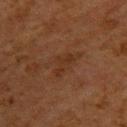| feature | finding |
|---|---|
| workup | catalogued during a skin exam; not biopsied |
| image | 15 mm crop, total-body photography |
| anatomic site | the upper back |
| subject | female, aged 48 to 52 |
| tile lighting | cross-polarized |
| diameter | about 3.5 mm |